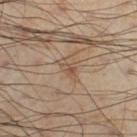A male patient in their mid- to late 50s.
A roughly 15 mm field-of-view crop from a total-body skin photograph.
Imaged with cross-polarized lighting.
The lesion is located on the leg.